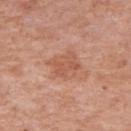follow-up: no biopsy performed (imaged during a skin exam)
body site: the arm
subject: female, aged 68 to 72
image: total-body-photography crop, ~15 mm field of view
lighting: white-light